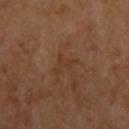Impression:
Part of a total-body skin-imaging series; this lesion was reviewed on a skin check and was not flagged for biopsy.
Background:
About 3.5 mm across. The lesion is located on the upper back. A lesion tile, about 15 mm wide, cut from a 3D total-body photograph. The tile uses cross-polarized illumination. The total-body-photography lesion software estimated an average lesion color of about L≈37 a*≈19 b*≈31 (CIELAB), a lesion–skin lightness drop of about 4, and a normalized border contrast of about 5. It also reported a border-irregularity index near 8.5/10, internal color variation of about 0 on a 0–10 scale, and radial color variation of about 0.